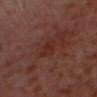Acquisition and patient details: Measured at roughly 3 mm in maximum diameter. The subject is a male aged 53–57. Automated tile analysis of the lesion measured an average lesion color of about L≈28 a*≈23 b*≈24 (CIELAB), about 5 CIELAB-L* units darker than the surrounding skin, and a normalized border contrast of about 5.5. And it measured a classifier nevus-likeness of about 0/100 and a detector confidence of about 100 out of 100 that the crop contains a lesion. A region of skin cropped from a whole-body photographic capture, roughly 15 mm wide. On the head or neck. Captured under cross-polarized illumination.